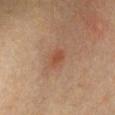The lesion was tiled from a total-body skin photograph and was not biopsied. Automated image analysis of the tile measured an average lesion color of about L≈43 a*≈22 b*≈29 (CIELAB), a lesion–skin lightness drop of about 7, and a lesion-to-skin contrast of about 6 (normalized; higher = more distinct). The tile uses cross-polarized illumination. From the chest. A male subject approximately 40 years of age. A region of skin cropped from a whole-body photographic capture, roughly 15 mm wide.